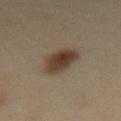A close-up tile cropped from a whole-body skin photograph, about 15 mm across. Automated tile analysis of the lesion measured a footprint of about 10 mm², a shape eccentricity near 0.7, and a shape-asymmetry score of about 0.15 (0 = symmetric). And it measured an average lesion color of about L≈31 a*≈12 b*≈22 (CIELAB), about 11 CIELAB-L* units darker than the surrounding skin, and a lesion-to-skin contrast of about 10.5 (normalized; higher = more distinct). The analysis additionally found a classifier nevus-likeness of about 100/100 and a detector confidence of about 100 out of 100 that the crop contains a lesion. Longest diameter approximately 4 mm. The lesion is located on the front of the torso. A female patient, in their mid-50s.